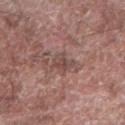biopsy_status: not biopsied; imaged during a skin examination
site: right forearm
lighting: white-light
patient:
  sex: male
  age_approx: 75
image:
  source: total-body photography crop
  field_of_view_mm: 15
automated_metrics:
  eccentricity: 0.7
  shape_asymmetry: 0.35
  color_variation_0_10: 3.0